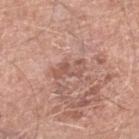Impression:
Imaged during a routine full-body skin examination; the lesion was not biopsied and no histopathology is available.
Acquisition and patient details:
The recorded lesion diameter is about 4 mm. The tile uses white-light illumination. The subject is a male aged approximately 80. From the leg. A roughly 15 mm field-of-view crop from a total-body skin photograph. Automated image analysis of the tile measured border irregularity of about 5.5 on a 0–10 scale and a color-variation rating of about 3/10. And it measured an automated nevus-likeness rating near 0 out of 100.